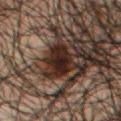<case>
  <biopsy_status>not biopsied; imaged during a skin examination</biopsy_status>
  <site>mid back</site>
  <patient>
    <sex>male</sex>
    <age_approx>50</age_approx>
  </patient>
  <lesion_size>
    <long_diameter_mm_approx>4.0</long_diameter_mm_approx>
  </lesion_size>
  <image>
    <source>total-body photography crop</source>
    <field_of_view_mm>15</field_of_view_mm>
  </image>
  <lighting>cross-polarized</lighting>
</case>